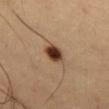Assessment:
Captured during whole-body skin photography for melanoma surveillance; the lesion was not biopsied.
Clinical summary:
Automated image analysis of the tile measured a footprint of about 6 mm², an outline eccentricity of about 0.55 (0 = round, 1 = elongated), and two-axis asymmetry of about 0.1. The software also gave a mean CIELAB color near L≈39 a*≈19 b*≈29, roughly 18 lightness units darker than nearby skin, and a normalized lesion–skin contrast near 13.5. And it measured border irregularity of about 1 on a 0–10 scale and peripheral color asymmetry of about 1.5. Measured at roughly 3 mm in maximum diameter. The patient is a female aged 48–52. Imaged with cross-polarized lighting. On the abdomen. A 15 mm close-up extracted from a 3D total-body photography capture.